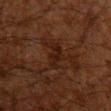Case summary:
– follow-up: imaged on a skin check; not biopsied
– subject: male, aged 58 to 62
– diameter: ≈3.5 mm
– image source: 15 mm crop, total-body photography
– lighting: cross-polarized
– site: the chest